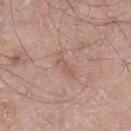The lesion was photographed on a routine skin check and not biopsied; there is no pathology result. About 3 mm across. A 15 mm close-up tile from a total-body photography series done for melanoma screening. The subject is a male roughly 70 years of age. The tile uses white-light illumination. From the left thigh. Automated tile analysis of the lesion measured a lesion area of about 3 mm², an outline eccentricity of about 0.95 (0 = round, 1 = elongated), and two-axis asymmetry of about 0.4. And it measured an automated nevus-likeness rating near 0 out of 100 and lesion-presence confidence of about 100/100.